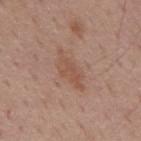– biopsy status — no biopsy performed (imaged during a skin exam)
– body site — the mid back
– patient — male, aged around 60
– acquisition — total-body-photography crop, ~15 mm field of view
– illumination — white-light illumination